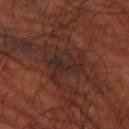- follow-up: no biopsy performed (imaged during a skin exam)
- subject: male, roughly 70 years of age
- image: ~15 mm tile from a whole-body skin photo
- site: the left thigh